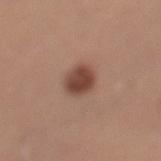notes=imaged on a skin check; not biopsied | body site=the left lower leg | image source=~15 mm crop, total-body skin-cancer survey | patient=female, in their mid-30s.